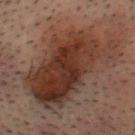Findings:
- follow-up: imaged on a skin check; not biopsied
- patient: male, aged around 30
- illumination: cross-polarized
- diameter: ~11.5 mm (longest diameter)
- body site: the head or neck
- acquisition: total-body-photography crop, ~15 mm field of view
- image-analysis metrics: an average lesion color of about L≈28 a*≈16 b*≈21 (CIELAB), a lesion–skin lightness drop of about 9, and a normalized border contrast of about 9.5; a border-irregularity index near 4.5/10 and peripheral color asymmetry of about 2; a classifier nevus-likeness of about 5/100 and a detector confidence of about 100 out of 100 that the crop contains a lesion